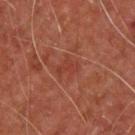The lesion was photographed on a routine skin check and not biopsied; there is no pathology result.
Measured at roughly 2.5 mm in maximum diameter.
The subject is a male approximately 65 years of age.
Automated image analysis of the tile measured an average lesion color of about L≈35 a*≈27 b*≈28 (CIELAB), about 5 CIELAB-L* units darker than the surrounding skin, and a normalized lesion–skin contrast near 4.5. The software also gave a classifier nevus-likeness of about 0/100 and lesion-presence confidence of about 100/100.
This is a cross-polarized tile.
From the back.
A 15 mm crop from a total-body photograph taken for skin-cancer surveillance.